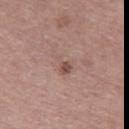Q: Was this lesion biopsied?
A: no biopsy performed (imaged during a skin exam)
Q: How large is the lesion?
A: ≈4 mm
Q: What did automated image analysis measure?
A: a footprint of about 6.5 mm², a shape eccentricity near 0.75, and a shape-asymmetry score of about 0.35 (0 = symmetric); a border-irregularity rating of about 4/10 and a color-variation rating of about 7/10; a detector confidence of about 100 out of 100 that the crop contains a lesion
Q: Patient demographics?
A: female, in their 40s
Q: Where on the body is the lesion?
A: the left thigh
Q: How was this image acquired?
A: ~15 mm crop, total-body skin-cancer survey